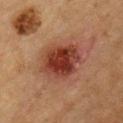The lesion was tiled from a total-body skin photograph and was not biopsied. The lesion's longest dimension is about 5.5 mm. Imaged with cross-polarized lighting. The total-body-photography lesion software estimated a footprint of about 19 mm² and two-axis asymmetry of about 0.15. It also reported an average lesion color of about L≈33 a*≈23 b*≈26 (CIELAB), roughly 12 lightness units darker than nearby skin, and a lesion-to-skin contrast of about 10.5 (normalized; higher = more distinct). It also reported peripheral color asymmetry of about 1.5. And it measured lesion-presence confidence of about 100/100. A region of skin cropped from a whole-body photographic capture, roughly 15 mm wide. The lesion is located on the chest. A male subject roughly 60 years of age.